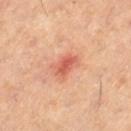Findings:
* notes — imaged on a skin check; not biopsied
* lesion size — ~3 mm (longest diameter)
* image source — 15 mm crop, total-body photography
* location — the left thigh
* subject — female, aged 38 to 42